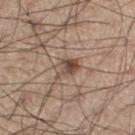| feature | finding |
|---|---|
| biopsy status | total-body-photography surveillance lesion; no biopsy |
| anatomic site | the leg |
| image | 15 mm crop, total-body photography |
| patient | male, aged 53–57 |
| diameter | about 3.5 mm |